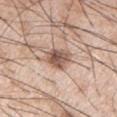This lesion was catalogued during total-body skin photography and was not selected for biopsy. A 15 mm close-up extracted from a 3D total-body photography capture. The recorded lesion diameter is about 3.5 mm. A male subject aged 53 to 57. The lesion is on the left upper arm. This is a white-light tile.